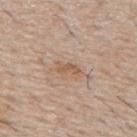{
  "biopsy_status": "not biopsied; imaged during a skin examination",
  "site": "mid back",
  "lesion_size": {
    "long_diameter_mm_approx": 3.0
  },
  "patient": {
    "sex": "male",
    "age_approx": 55
  },
  "image": {
    "source": "total-body photography crop",
    "field_of_view_mm": 15
  }
}